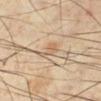Part of a total-body skin-imaging series; this lesion was reviewed on a skin check and was not flagged for biopsy. Captured under cross-polarized illumination. A male subject aged around 55. Located on the right lower leg. The total-body-photography lesion software estimated roughly 8 lightness units darker than nearby skin and a normalized lesion–skin contrast near 5.5. A close-up tile cropped from a whole-body skin photograph, about 15 mm across.